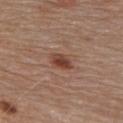biopsy_status: not biopsied; imaged during a skin examination
patient:
  sex: male
  age_approx: 80
site: chest
image:
  source: total-body photography crop
  field_of_view_mm: 15